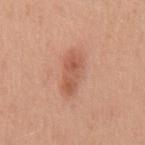Captured during whole-body skin photography for melanoma surveillance; the lesion was not biopsied.
Captured under white-light illumination.
A 15 mm crop from a total-body photograph taken for skin-cancer surveillance.
On the mid back.
The lesion's longest dimension is about 5 mm.
A male patient in their 50s.